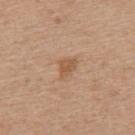Q: How large is the lesion?
A: ~3 mm (longest diameter)
Q: Who is the patient?
A: female, aged 43–47
Q: What kind of image is this?
A: 15 mm crop, total-body photography
Q: How was the tile lit?
A: white-light illumination
Q: What is the anatomic site?
A: the upper back